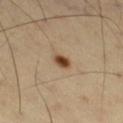This lesion was catalogued during total-body skin photography and was not selected for biopsy. A male subject, approximately 55 years of age. Measured at roughly 2 mm in maximum diameter. A 15 mm close-up tile from a total-body photography series done for melanoma screening. Captured under cross-polarized illumination. The lesion is on the right thigh.